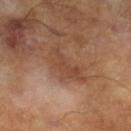workup: no biopsy performed (imaged during a skin exam)
lighting: cross-polarized
imaging modality: 15 mm crop, total-body photography
size: ≈5 mm
patient: male, aged around 65
TBP lesion metrics: an area of roughly 8 mm² and two-axis asymmetry of about 0.45; radial color variation of about 1